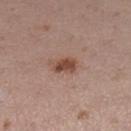| field | value |
|---|---|
| notes | no biopsy performed (imaged during a skin exam) |
| patient | female, about 30 years old |
| site | the right lower leg |
| acquisition | total-body-photography crop, ~15 mm field of view |
| lesion diameter | about 3.5 mm |
| illumination | white-light illumination |
| automated metrics | an area of roughly 5 mm² and an outline eccentricity of about 0.8 (0 = round, 1 = elongated); an average lesion color of about L≈47 a*≈21 b*≈27 (CIELAB) |